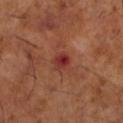Part of a total-body skin-imaging series; this lesion was reviewed on a skin check and was not flagged for biopsy.
The lesion is located on the left lower leg.
The lesion's longest dimension is about 2.5 mm.
A male patient, aged 63 to 67.
A 15 mm crop from a total-body photograph taken for skin-cancer surveillance.
Automated image analysis of the tile measured a nevus-likeness score of about 10/100.